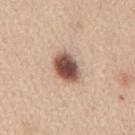| feature | finding |
|---|---|
| workup | imaged on a skin check; not biopsied |
| image | 15 mm crop, total-body photography |
| body site | the mid back |
| automated metrics | a footprint of about 9.5 mm², a shape eccentricity near 0.75, and a symmetry-axis asymmetry near 0.15; about 20 CIELAB-L* units darker than the surrounding skin and a lesion-to-skin contrast of about 13 (normalized; higher = more distinct); an automated nevus-likeness rating near 100 out of 100 and a lesion-detection confidence of about 100/100 |
| patient | female, about 40 years old |
| size | ≈4 mm |